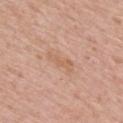Background:
The lesion is located on the upper back. A 15 mm crop from a total-body photograph taken for skin-cancer surveillance. The subject is a female about 60 years old. Measured at roughly 3.5 mm in maximum diameter.